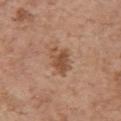biopsy_status: not biopsied; imaged during a skin examination
automated_metrics:
  color_variation_0_10: 4.5
  nevus_likeness_0_100: 55
  lesion_detection_confidence_0_100: 100
lesion_size:
  long_diameter_mm_approx: 4.5
patient:
  sex: female
  age_approx: 65
image:
  source: total-body photography crop
  field_of_view_mm: 15
site: left upper arm
lighting: white-light